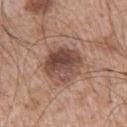Clinical impression: Recorded during total-body skin imaging; not selected for excision or biopsy. Clinical summary: A male subject in their mid-60s. This image is a 15 mm lesion crop taken from a total-body photograph. The lesion is located on the left upper arm.